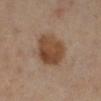Recorded during total-body skin imaging; not selected for excision or biopsy. Measured at roughly 5 mm in maximum diameter. On the right lower leg. A female subject, aged 43 to 47. A 15 mm close-up extracted from a 3D total-body photography capture. An algorithmic analysis of the crop reported a lesion area of about 17 mm², a shape eccentricity near 0.5, and a shape-asymmetry score of about 0.15 (0 = symmetric). And it measured about 11 CIELAB-L* units darker than the surrounding skin. And it measured border irregularity of about 1.5 on a 0–10 scale and a within-lesion color-variation index near 4.5/10. The analysis additionally found a nevus-likeness score of about 95/100 and a detector confidence of about 100 out of 100 that the crop contains a lesion.